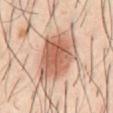| key | value |
|---|---|
| notes | imaged on a skin check; not biopsied |
| diameter | ~7.5 mm (longest diameter) |
| location | the abdomen |
| subject | male, aged approximately 40 |
| tile lighting | cross-polarized illumination |
| acquisition | total-body-photography crop, ~15 mm field of view |